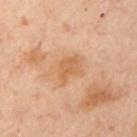biopsy status = catalogued during a skin exam; not biopsied | patient = female, aged 53 to 57 | automated lesion analysis = an average lesion color of about L≈50 a*≈19 b*≈33 (CIELAB) and roughly 6 lightness units darker than nearby skin; border irregularity of about 3 on a 0–10 scale, a color-variation rating of about 1.5/10, and peripheral color asymmetry of about 0.5; an automated nevus-likeness rating near 0 out of 100 and a detector confidence of about 100 out of 100 that the crop contains a lesion | lesion diameter = ≈3 mm | acquisition = 15 mm crop, total-body photography | body site = the front of the torso | illumination = cross-polarized illumination.